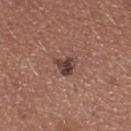Impression:
The lesion was photographed on a routine skin check and not biopsied; there is no pathology result.
Image and clinical context:
Longest diameter approximately 2.5 mm. The lesion is on the upper back. A female patient aged approximately 25. Cropped from a whole-body photographic skin survey; the tile spans about 15 mm.